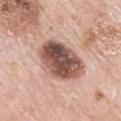Captured during whole-body skin photography for melanoma surveillance; the lesion was not biopsied. The recorded lesion diameter is about 6 mm. The lesion is on the mid back. A roughly 15 mm field-of-view crop from a total-body skin photograph. A male subject roughly 80 years of age. The tile uses white-light illumination. Automated image analysis of the tile measured a lesion color around L≈52 a*≈21 b*≈25 in CIELAB, a lesion–skin lightness drop of about 19, and a lesion-to-skin contrast of about 12 (normalized; higher = more distinct). The software also gave border irregularity of about 1.5 on a 0–10 scale and a within-lesion color-variation index near 9.5/10.